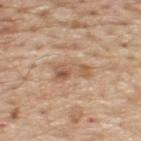Q: Was this lesion biopsied?
A: no biopsy performed (imaged during a skin exam)
Q: Lesion size?
A: ~4.5 mm (longest diameter)
Q: What is the imaging modality?
A: total-body-photography crop, ~15 mm field of view
Q: Automated lesion metrics?
A: a footprint of about 8.5 mm², an eccentricity of roughly 0.85, and a symmetry-axis asymmetry near 0.4
Q: What are the patient's age and sex?
A: male, approximately 70 years of age
Q: What lighting was used for the tile?
A: white-light
Q: Where on the body is the lesion?
A: the upper back